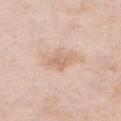About 3 mm across. The total-body-photography lesion software estimated a lesion area of about 5.5 mm², an eccentricity of roughly 0.75, and two-axis asymmetry of about 0.35. It also reported an average lesion color of about L≈67 a*≈18 b*≈30 (CIELAB), roughly 8 lightness units darker than nearby skin, and a normalized border contrast of about 5.5. And it measured an automated nevus-likeness rating near 0 out of 100. A 15 mm close-up extracted from a 3D total-body photography capture. A female patient, aged approximately 75. The lesion is on the chest. This is a white-light tile.